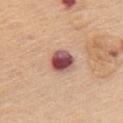Recorded during total-body skin imaging; not selected for excision or biopsy.
A lesion tile, about 15 mm wide, cut from a 3D total-body photograph.
A female patient in their mid- to late 60s.
Measured at roughly 3.5 mm in maximum diameter.
The lesion is on the chest.
Captured under white-light illumination.